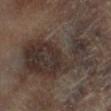Impression: The lesion was tiled from a total-body skin photograph and was not biopsied. Image and clinical context: Automated image analysis of the tile measured roughly 8 lightness units darker than nearby skin and a normalized border contrast of about 8.5. The analysis additionally found a nevus-likeness score of about 15/100 and a detector confidence of about 35 out of 100 that the crop contains a lesion. The lesion is on the left lower leg. The subject is a male in their mid- to late 60s. Cropped from a total-body skin-imaging series; the visible field is about 15 mm.